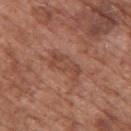Recorded during total-body skin imaging; not selected for excision or biopsy. The lesion is located on the upper back. The total-body-photography lesion software estimated a mean CIELAB color near L≈46 a*≈23 b*≈28, a lesion–skin lightness drop of about 7, and a normalized lesion–skin contrast near 5.5. And it measured an automated nevus-likeness rating near 0 out of 100. A male subject aged 73–77. A 15 mm close-up extracted from a 3D total-body photography capture.